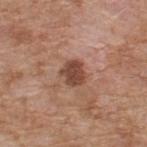<tbp_lesion>
  <biopsy_status>not biopsied; imaged during a skin examination</biopsy_status>
</tbp_lesion>